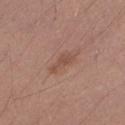Findings:
- lighting: white-light
- imaging modality: total-body-photography crop, ~15 mm field of view
- diameter: about 3 mm
- automated lesion analysis: an area of roughly 3.5 mm², an outline eccentricity of about 0.9 (0 = round, 1 = elongated), and a shape-asymmetry score of about 0.45 (0 = symmetric); a mean CIELAB color near L≈50 a*≈20 b*≈27 and a lesion–skin lightness drop of about 8; a border-irregularity index near 5/10, internal color variation of about 0.5 on a 0–10 scale, and peripheral color asymmetry of about 0
- patient: male, about 25 years old
- site: the right forearm